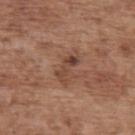Part of a total-body skin-imaging series; this lesion was reviewed on a skin check and was not flagged for biopsy. A roughly 15 mm field-of-view crop from a total-body skin photograph. A male patient aged around 70. On the upper back.